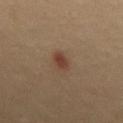{"biopsy_status": "not biopsied; imaged during a skin examination"}